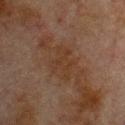workup — imaged on a skin check; not biopsied | site — the chest | size — ~3.5 mm (longest diameter) | patient — male, approximately 80 years of age | imaging modality — total-body-photography crop, ~15 mm field of view | tile lighting — cross-polarized.